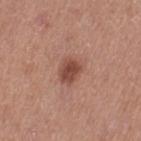{
  "biopsy_status": "not biopsied; imaged during a skin examination",
  "site": "left thigh",
  "lesion_size": {
    "long_diameter_mm_approx": 3.0
  },
  "image": {
    "source": "total-body photography crop",
    "field_of_view_mm": 15
  },
  "patient": {
    "sex": "female",
    "age_approx": 40
  },
  "lighting": "white-light"
}